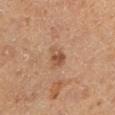{"biopsy_status": "not biopsied; imaged during a skin examination", "image": {"source": "total-body photography crop", "field_of_view_mm": 15}, "lesion_size": {"long_diameter_mm_approx": 2.5}, "lighting": "cross-polarized", "site": "left lower leg", "patient": {"sex": "male", "age_approx": 60}}